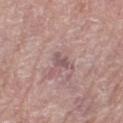The lesion was tiled from a total-body skin photograph and was not biopsied.